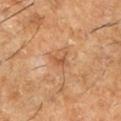follow-up: imaged on a skin check; not biopsied
subject: male, aged around 60
location: the left lower leg
image source: ~15 mm crop, total-body skin-cancer survey